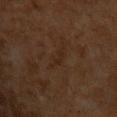workup = no biopsy performed (imaged during a skin exam) | image = total-body-photography crop, ~15 mm field of view | subject = male, aged around 60 | site = the upper back.